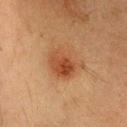Notes:
* biopsy status — no biopsy performed (imaged during a skin exam)
* automated lesion analysis — a footprint of about 11 mm², an eccentricity of roughly 0.7, and two-axis asymmetry of about 0.25; border irregularity of about 3 on a 0–10 scale, internal color variation of about 6 on a 0–10 scale, and peripheral color asymmetry of about 2
* site — the head or neck
* size — ~4.5 mm (longest diameter)
* imaging modality — ~15 mm crop, total-body skin-cancer survey
* subject — female, about 20 years old
* illumination — cross-polarized